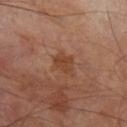No biopsy was performed on this lesion — it was imaged during a full skin examination and was not determined to be concerning. About 3 mm across. The tile uses cross-polarized illumination. From the right lower leg. A close-up tile cropped from a whole-body skin photograph, about 15 mm across. A male patient aged around 70.